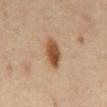Part of a total-body skin-imaging series; this lesion was reviewed on a skin check and was not flagged for biopsy. A male subject roughly 55 years of age. A 15 mm crop from a total-body photograph taken for skin-cancer surveillance. About 4 mm across. An algorithmic analysis of the crop reported a lesion area of about 7 mm² and an outline eccentricity of about 0.8 (0 = round, 1 = elongated). The analysis additionally found a lesion color around L≈49 a*≈20 b*≈34 in CIELAB, roughly 14 lightness units darker than nearby skin, and a normalized lesion–skin contrast near 10.5. The analysis additionally found a border-irregularity index near 2/10, a color-variation rating of about 5/10, and radial color variation of about 1.5. And it measured a nevus-likeness score of about 100/100 and lesion-presence confidence of about 100/100. The tile uses cross-polarized illumination. From the abdomen.